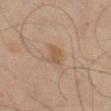This lesion was catalogued during total-body skin photography and was not selected for biopsy.
Longest diameter approximately 2.5 mm.
The subject is a male roughly 65 years of age.
The lesion is located on the left thigh.
A region of skin cropped from a whole-body photographic capture, roughly 15 mm wide.
This is a cross-polarized tile.
Automated tile analysis of the lesion measured a lesion area of about 4 mm² and an eccentricity of roughly 0.7.